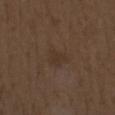Imaged during a routine full-body skin examination; the lesion was not biopsied and no histopathology is available. A roughly 15 mm field-of-view crop from a total-body skin photograph. From the left forearm. The recorded lesion diameter is about 3 mm. This is a white-light tile. The total-body-photography lesion software estimated a lesion area of about 4.5 mm², a shape eccentricity near 0.55, and a shape-asymmetry score of about 0.45 (0 = symmetric). The software also gave a lesion color around L≈32 a*≈13 b*≈24 in CIELAB. A male patient, aged 68 to 72.